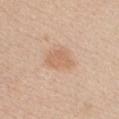Assessment: Recorded during total-body skin imaging; not selected for excision or biopsy. Clinical summary: From the chest. About 3.5 mm across. A close-up tile cropped from a whole-body skin photograph, about 15 mm across. The total-body-photography lesion software estimated an average lesion color of about L≈64 a*≈20 b*≈33 (CIELAB), a lesion–skin lightness drop of about 8, and a lesion-to-skin contrast of about 5.5 (normalized; higher = more distinct). A female subject aged approximately 40.